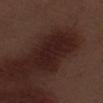Captured during whole-body skin photography for melanoma surveillance; the lesion was not biopsied. Longest diameter approximately 5.5 mm. The subject is a male aged approximately 70. Cropped from a whole-body photographic skin survey; the tile spans about 15 mm. The lesion is located on the left thigh.